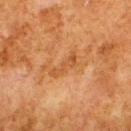Q: Was a biopsy performed?
A: total-body-photography surveillance lesion; no biopsy
Q: What is the anatomic site?
A: the upper back
Q: What are the patient's age and sex?
A: male, aged around 80
Q: What is the imaging modality?
A: ~15 mm tile from a whole-body skin photo